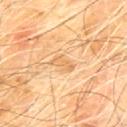{
  "site": "chest",
  "lesion_size": {
    "long_diameter_mm_approx": 3.0
  },
  "lighting": "cross-polarized",
  "automated_metrics": {
    "nevus_likeness_0_100": 0,
    "lesion_detection_confidence_0_100": 100
  },
  "image": {
    "source": "total-body photography crop",
    "field_of_view_mm": 15
  },
  "patient": {
    "sex": "male",
    "age_approx": 60
  }
}